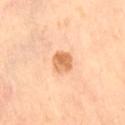workup=imaged on a skin check; not biopsied
imaging modality=15 mm crop, total-body photography
location=the right thigh
TBP lesion metrics=an area of roughly 6 mm² and two-axis asymmetry of about 0.15; roughly 13 lightness units darker than nearby skin; a border-irregularity index near 1.5/10 and a color-variation rating of about 4.5/10; a classifier nevus-likeness of about 85/100 and a lesion-detection confidence of about 100/100
subject=female, approximately 55 years of age